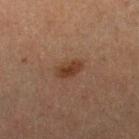Background: A roughly 15 mm field-of-view crop from a total-body skin photograph. The lesion is located on the leg. Approximately 3 mm at its widest. This is a cross-polarized tile. The patient is a male in their 30s.